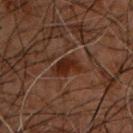Recorded during total-body skin imaging; not selected for excision or biopsy. The lesion is located on the chest. Cropped from a whole-body photographic skin survey; the tile spans about 15 mm. A male patient aged around 50. About 4.5 mm across. Captured under cross-polarized illumination.